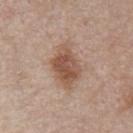{
  "biopsy_status": "not biopsied; imaged during a skin examination",
  "patient": {
    "sex": "male",
    "age_approx": 55
  },
  "lighting": "white-light",
  "image": {
    "source": "total-body photography crop",
    "field_of_view_mm": 15
  },
  "lesion_size": {
    "long_diameter_mm_approx": 5.0
  },
  "site": "chest"
}